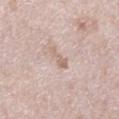Q: Was a biopsy performed?
A: catalogued during a skin exam; not biopsied
Q: What is the imaging modality?
A: total-body-photography crop, ~15 mm field of view
Q: Patient demographics?
A: male, roughly 60 years of age
Q: Where on the body is the lesion?
A: the right lower leg